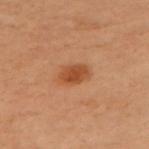Recorded during total-body skin imaging; not selected for excision or biopsy. On the front of the torso. The patient is a female approximately 60 years of age. This image is a 15 mm lesion crop taken from a total-body photograph.